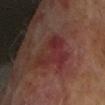This lesion was catalogued during total-body skin photography and was not selected for biopsy. The patient is a female aged approximately 75. Captured under cross-polarized illumination. A 15 mm close-up extracted from a 3D total-body photography capture. The lesion is on the arm. The recorded lesion diameter is about 5 mm.